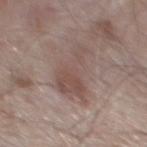<record>
<biopsy_status>not biopsied; imaged during a skin examination</biopsy_status>
<image>
  <source>total-body photography crop</source>
  <field_of_view_mm>15</field_of_view_mm>
</image>
<patient>
  <sex>male</sex>
  <age_approx>55</age_approx>
</patient>
<site>back</site>
</record>